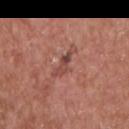Clinical impression: No biopsy was performed on this lesion — it was imaged during a full skin examination and was not determined to be concerning. Background: The lesion is on the left upper arm. Longest diameter approximately 3 mm. A region of skin cropped from a whole-body photographic capture, roughly 15 mm wide. Captured under white-light illumination. A male patient roughly 65 years of age.